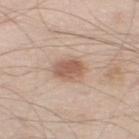Clinical impression:
This lesion was catalogued during total-body skin photography and was not selected for biopsy.
Clinical summary:
A male subject, in their 60s. On the left thigh. A roughly 15 mm field-of-view crop from a total-body skin photograph.